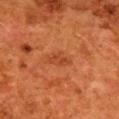<lesion>
<biopsy_status>not biopsied; imaged during a skin examination</biopsy_status>
<image>
  <source>total-body photography crop</source>
  <field_of_view_mm>15</field_of_view_mm>
</image>
<lighting>cross-polarized</lighting>
<site>upper back</site>
<lesion_size>
  <long_diameter_mm_approx>2.5</long_diameter_mm_approx>
</lesion_size>
<automated_metrics>
  <area_mm2_approx>2.5</area_mm2_approx>
  <eccentricity>0.9</eccentricity>
  <shape_asymmetry>0.45</shape_asymmetry>
  <cielab_L>37</cielab_L>
  <cielab_a>26</cielab_a>
  <cielab_b>35</cielab_b>
  <vs_skin_contrast_norm>5.5</vs_skin_contrast_norm>
  <border_irregularity_0_10>4.5</border_irregularity_0_10>
  <color_variation_0_10>0.0</color_variation_0_10>
  <peripheral_color_asymmetry>0.0</peripheral_color_asymmetry>
  <nevus_likeness_0_100>10</nevus_likeness_0_100>
  <lesion_detection_confidence_0_100>100</lesion_detection_confidence_0_100>
</automated_metrics>
<patient>
  <sex>female</sex>
  <age_approx>50</age_approx>
</patient>
</lesion>